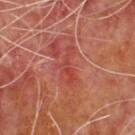Clinical impression: Captured during whole-body skin photography for melanoma surveillance; the lesion was not biopsied. Background: This is a cross-polarized tile. The lesion-visualizer software estimated a mean CIELAB color near L≈44 a*≈33 b*≈30 and roughly 7 lightness units darker than nearby skin. And it measured a border-irregularity rating of about 7.5/10, a color-variation rating of about 3.5/10, and peripheral color asymmetry of about 1. It also reported a nevus-likeness score of about 0/100 and a lesion-detection confidence of about 95/100. About 4.5 mm across. A male subject approximately 60 years of age. This image is a 15 mm lesion crop taken from a total-body photograph. The lesion is on the upper back.